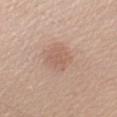Captured during whole-body skin photography for melanoma surveillance; the lesion was not biopsied.